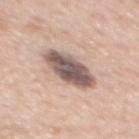The lesion was photographed on a routine skin check and not biopsied; there is no pathology result.
The tile uses white-light illumination.
An algorithmic analysis of the crop reported a lesion area of about 15 mm² and a shape-asymmetry score of about 0.1 (0 = symmetric).
Approximately 6 mm at its widest.
A male patient, aged approximately 60.
On the mid back.
A region of skin cropped from a whole-body photographic capture, roughly 15 mm wide.